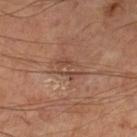Q: Is there a histopathology result?
A: total-body-photography surveillance lesion; no biopsy
Q: What is the anatomic site?
A: the left lower leg
Q: What kind of image is this?
A: ~15 mm crop, total-body skin-cancer survey
Q: What is the lesion's diameter?
A: ~3.5 mm (longest diameter)
Q: What lighting was used for the tile?
A: cross-polarized
Q: What are the patient's age and sex?
A: male, aged approximately 70